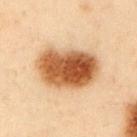follow-up: total-body-photography surveillance lesion; no biopsy | illumination: cross-polarized | patient: male, in their mid-50s | size: about 7.5 mm | anatomic site: the left upper arm | image: ~15 mm tile from a whole-body skin photo.